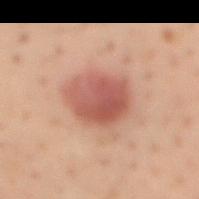Recorded during total-body skin imaging; not selected for excision or biopsy.
Longest diameter approximately 5 mm.
The lesion-visualizer software estimated an area of roughly 16 mm² and an outline eccentricity of about 0.5 (0 = round, 1 = elongated). The software also gave a lesion–skin lightness drop of about 10. It also reported border irregularity of about 1.5 on a 0–10 scale and radial color variation of about 1.5.
A male subject, aged approximately 40.
A 15 mm crop from a total-body photograph taken for skin-cancer surveillance.
Imaged with cross-polarized lighting.
From the mid back.